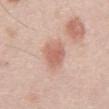biopsy_status: not biopsied; imaged during a skin examination
lighting: white-light
lesion_size:
  long_diameter_mm_approx: 3.5
site: abdomen
image:
  source: total-body photography crop
  field_of_view_mm: 15
automated_metrics:
  area_mm2_approx: 8.0
  shape_asymmetry: 0.2
  cielab_L: 62
  cielab_a: 23
  cielab_b: 28
  vs_skin_darker_L: 10.0
  vs_skin_contrast_norm: 6.5
  border_irregularity_0_10: 2.0
  peripheral_color_asymmetry: 1.0
patient:
  sex: male
  age_approx: 50